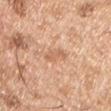Clinical impression:
Captured during whole-body skin photography for melanoma surveillance; the lesion was not biopsied.
Clinical summary:
Longest diameter approximately 3 mm. A roughly 15 mm field-of-view crop from a total-body skin photograph. On the left upper arm. A male subject, in their mid-50s. Captured under white-light illumination.